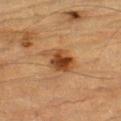Clinical summary:
A lesion tile, about 15 mm wide, cut from a 3D total-body photograph. The lesion-visualizer software estimated an area of roughly 9 mm², an eccentricity of roughly 0.75, and two-axis asymmetry of about 0.3. The analysis additionally found a mean CIELAB color near L≈40 a*≈21 b*≈34 and a normalized border contrast of about 9.5. The tile uses cross-polarized illumination. A male patient aged approximately 85 or older. The lesion's longest dimension is about 4 mm. On the left thigh.